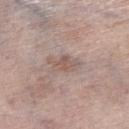{
  "biopsy_status": "not biopsied; imaged during a skin examination",
  "site": "left lower leg",
  "patient": {
    "sex": "female",
    "age_approx": 65
  },
  "image": {
    "source": "total-body photography crop",
    "field_of_view_mm": 15
  },
  "lighting": "white-light",
  "lesion_size": {
    "long_diameter_mm_approx": 4.0
  }
}